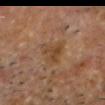biopsy status — imaged on a skin check; not biopsied
automated metrics — a footprint of about 7.5 mm² and a symmetry-axis asymmetry near 0.55; an average lesion color of about L≈41 a*≈17 b*≈31 (CIELAB), about 6 CIELAB-L* units darker than the surrounding skin, and a normalized lesion–skin contrast near 6.5
subject — male, about 65 years old
location — the head or neck
lesion size — about 4 mm
imaging modality — 15 mm crop, total-body photography